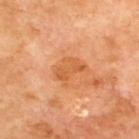Captured during whole-body skin photography for melanoma surveillance; the lesion was not biopsied.
The lesion is located on the upper back.
A lesion tile, about 15 mm wide, cut from a 3D total-body photograph.
The patient is a male aged 68–72.